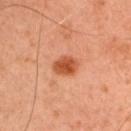The lesion was photographed on a routine skin check and not biopsied; there is no pathology result. The subject is a male about 45 years old. About 3 mm across. Captured under cross-polarized illumination. A 15 mm close-up tile from a total-body photography series done for melanoma screening. Located on the left upper arm. An algorithmic analysis of the crop reported a mean CIELAB color near L≈50 a*≈29 b*≈38 and a normalized lesion–skin contrast near 9. The software also gave peripheral color asymmetry of about 1. It also reported a nevus-likeness score of about 100/100 and a detector confidence of about 100 out of 100 that the crop contains a lesion.